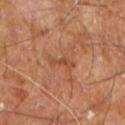follow-up: total-body-photography surveillance lesion; no biopsy
lesion diameter: ≈2.5 mm
subject: male, roughly 60 years of age
imaging modality: 15 mm crop, total-body photography
anatomic site: the right leg